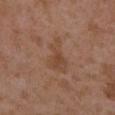location: the left upper arm | acquisition: total-body-photography crop, ~15 mm field of view | lighting: white-light illumination | size: ≈5 mm | subject: female, in their mid- to late 30s | TBP lesion metrics: a shape eccentricity near 0.9 and two-axis asymmetry of about 0.55; an average lesion color of about L≈45 a*≈19 b*≈30 (CIELAB) and a normalized lesion–skin contrast near 5.5; a border-irregularity index near 6/10, a color-variation rating of about 2/10, and radial color variation of about 0.5; an automated nevus-likeness rating near 0 out of 100 and a detector confidence of about 100 out of 100 that the crop contains a lesion.